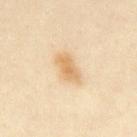No biopsy was performed on this lesion — it was imaged during a full skin examination and was not determined to be concerning. The total-body-photography lesion software estimated a lesion area of about 7 mm², an eccentricity of roughly 0.85, and a shape-asymmetry score of about 0.3 (0 = symmetric). It also reported a border-irregularity index near 3/10 and internal color variation of about 3 on a 0–10 scale. From the abdomen. A 15 mm crop from a total-body photograph taken for skin-cancer surveillance. About 4 mm across. The tile uses cross-polarized illumination. A male patient about 30 years old.